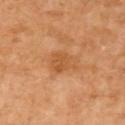Q: Was this lesion biopsied?
A: catalogued during a skin exam; not biopsied
Q: How large is the lesion?
A: ~3 mm (longest diameter)
Q: Who is the patient?
A: female, approximately 70 years of age
Q: Where on the body is the lesion?
A: the right upper arm
Q: What lighting was used for the tile?
A: cross-polarized illumination
Q: How was this image acquired?
A: 15 mm crop, total-body photography